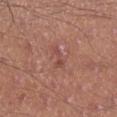<record>
<biopsy_status>not biopsied; imaged during a skin examination</biopsy_status>
<lighting>white-light</lighting>
<automated_metrics>
  <area_mm2_approx>2.5</area_mm2_approx>
  <eccentricity>0.9</eccentricity>
  <shape_asymmetry>0.45</shape_asymmetry>
  <cielab_L>48</cielab_L>
  <cielab_a>25</cielab_a>
  <cielab_b>25</cielab_b>
  <vs_skin_darker_L>7.0</vs_skin_darker_L>
  <vs_skin_contrast_norm>5.5</vs_skin_contrast_norm>
</automated_metrics>
<patient>
  <sex>male</sex>
  <age_approx>45</age_approx>
</patient>
<image>
  <source>total-body photography crop</source>
  <field_of_view_mm>15</field_of_view_mm>
</image>
<site>leg</site>
<lesion_size>
  <long_diameter_mm_approx>2.5</long_diameter_mm_approx>
</lesion_size>
</record>